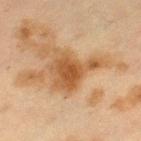Impression:
The lesion was tiled from a total-body skin photograph and was not biopsied.
Clinical summary:
From the leg. A female subject, approximately 40 years of age. This image is a 15 mm lesion crop taken from a total-body photograph. This is a cross-polarized tile. Measured at roughly 6 mm in maximum diameter.